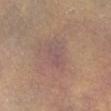Background:
On the chest. This is a white-light tile. A female subject, aged around 50. Cropped from a whole-body photographic skin survey; the tile spans about 15 mm.